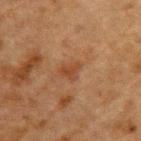workup=catalogued during a skin exam; not biopsied | anatomic site=the back | size=≈2.5 mm | subject=male, about 50 years old | lighting=cross-polarized | acquisition=total-body-photography crop, ~15 mm field of view.